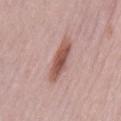No biopsy was performed on this lesion — it was imaged during a full skin examination and was not determined to be concerning.